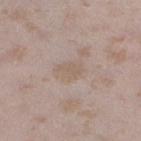This lesion was catalogued during total-body skin photography and was not selected for biopsy. Imaged with white-light lighting. A female subject in their mid-20s. About 3 mm across. A region of skin cropped from a whole-body photographic capture, roughly 15 mm wide. The lesion-visualizer software estimated an average lesion color of about L≈59 a*≈13 b*≈25 (CIELAB) and a normalized lesion–skin contrast near 4.5. And it measured an automated nevus-likeness rating near 0 out of 100 and lesion-presence confidence of about 100/100. The lesion is located on the left lower leg.